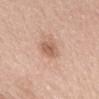| feature | finding |
|---|---|
| follow-up | total-body-photography surveillance lesion; no biopsy |
| image source | 15 mm crop, total-body photography |
| patient | female, in their 60s |
| tile lighting | white-light illumination |
| image-analysis metrics | a mean CIELAB color near L≈60 a*≈21 b*≈30; internal color variation of about 2.5 on a 0–10 scale and a peripheral color-asymmetry measure near 1; an automated nevus-likeness rating near 65 out of 100 |
| size | ≈3 mm |
| site | the mid back |